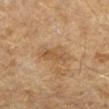biopsy_status: not biopsied; imaged during a skin examination
image:
  source: total-body photography crop
  field_of_view_mm: 15
patient:
  sex: female
  age_approx: 60
site: arm